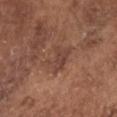Q: Was this lesion biopsied?
A: no biopsy performed (imaged during a skin exam)
Q: How was this image acquired?
A: 15 mm crop, total-body photography
Q: How large is the lesion?
A: about 4 mm
Q: What is the anatomic site?
A: the head or neck
Q: What lighting was used for the tile?
A: white-light
Q: What are the patient's age and sex?
A: male, aged 73–77